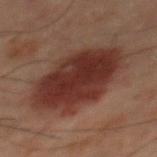Q: Was a biopsy performed?
A: no biopsy performed (imaged during a skin exam)
Q: Lesion size?
A: about 9 mm
Q: What is the imaging modality?
A: ~15 mm crop, total-body skin-cancer survey
Q: What did automated image analysis measure?
A: a footprint of about 34 mm², an eccentricity of roughly 0.8, and two-axis asymmetry of about 0.15; a lesion color around L≈23 a*≈18 b*≈19 in CIELAB, roughly 10 lightness units darker than nearby skin, and a normalized border contrast of about 11.5; a border-irregularity rating of about 2.5/10 and a color-variation rating of about 3.5/10; an automated nevus-likeness rating near 100 out of 100 and a detector confidence of about 100 out of 100 that the crop contains a lesion
Q: What is the anatomic site?
A: the back
Q: How was the tile lit?
A: cross-polarized
Q: Who is the patient?
A: male, in their 60s